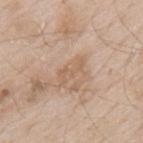Case summary:
• biopsy status · catalogued during a skin exam; not biopsied
• image-analysis metrics · an area of roughly 5.5 mm² and a shape eccentricity near 0.85; a lesion color around L≈61 a*≈16 b*≈31 in CIELAB, roughly 6 lightness units darker than nearby skin, and a normalized border contrast of about 5; a border-irregularity rating of about 5/10, a color-variation rating of about 3/10, and peripheral color asymmetry of about 1; a nevus-likeness score of about 0/100
• diameter · about 4 mm
• image source · 15 mm crop, total-body photography
• patient · male, in their mid- to late 60s
• location · the mid back